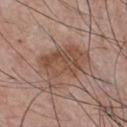Captured during whole-body skin photography for melanoma surveillance; the lesion was not biopsied. The lesion is on the chest. This image is a 15 mm lesion crop taken from a total-body photograph. The patient is a male aged approximately 55.